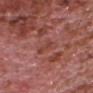Captured during whole-body skin photography for melanoma surveillance; the lesion was not biopsied. The total-body-photography lesion software estimated a footprint of about 4.5 mm² and an outline eccentricity of about 0.8 (0 = round, 1 = elongated). A close-up tile cropped from a whole-body skin photograph, about 15 mm across. The patient is a male roughly 75 years of age. From the head or neck. The recorded lesion diameter is about 3 mm.